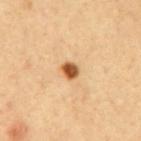Q: Is there a histopathology result?
A: no biopsy performed (imaged during a skin exam)